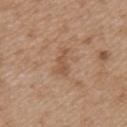Assessment:
Imaged during a routine full-body skin examination; the lesion was not biopsied and no histopathology is available.